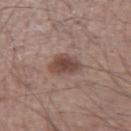This lesion was catalogued during total-body skin photography and was not selected for biopsy.
The tile uses white-light illumination.
Automated image analysis of the tile measured a shape eccentricity near 0.45 and a shape-asymmetry score of about 0.25 (0 = symmetric). The analysis additionally found border irregularity of about 2.5 on a 0–10 scale and peripheral color asymmetry of about 1.
The lesion is located on the right upper arm.
A male subject, aged 63 to 67.
Cropped from a whole-body photographic skin survey; the tile spans about 15 mm.
The lesion's longest dimension is about 3.5 mm.